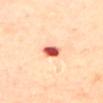<lesion>
  <lesion_size>
    <long_diameter_mm_approx>2.5</long_diameter_mm_approx>
  </lesion_size>
  <image>
    <source>total-body photography crop</source>
    <field_of_view_mm>15</field_of_view_mm>
  </image>
  <automated_metrics>
    <area_mm2_approx>4.0</area_mm2_approx>
    <shape_asymmetry>0.2</shape_asymmetry>
    <border_irregularity_0_10>2.0</border_irregularity_0_10>
    <color_variation_0_10>7.0</color_variation_0_10>
    <peripheral_color_asymmetry>2.5</peripheral_color_asymmetry>
    <nevus_likeness_0_100>0</nevus_likeness_0_100>
    <lesion_detection_confidence_0_100>100</lesion_detection_confidence_0_100>
  </automated_metrics>
  <patient>
    <sex>female</sex>
    <age_approx>60</age_approx>
  </patient>
  <site>upper back</site>
</lesion>